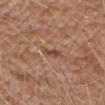biopsy status: imaged on a skin check; not biopsied | anatomic site: the left upper arm | illumination: white-light illumination | acquisition: 15 mm crop, total-body photography | patient: male, in their 60s | automated lesion analysis: an eccentricity of roughly 0.9 and a shape-asymmetry score of about 0.35 (0 = symmetric); a mean CIELAB color near L≈46 a*≈21 b*≈29, a lesion–skin lightness drop of about 10, and a normalized border contrast of about 7.5; a within-lesion color-variation index near 0.5/10 and radial color variation of about 0; a classifier nevus-likeness of about 0/100 and a lesion-detection confidence of about 95/100.